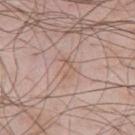notes: imaged on a skin check; not biopsied | body site: the chest | image source: ~15 mm tile from a whole-body skin photo | patient: male, aged approximately 55.